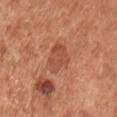No biopsy was performed on this lesion — it was imaged during a full skin examination and was not determined to be concerning. A region of skin cropped from a whole-body photographic capture, roughly 15 mm wide. Captured under white-light illumination. Measured at roughly 3.5 mm in maximum diameter. The total-body-photography lesion software estimated a mean CIELAB color near L≈51 a*≈27 b*≈34, roughly 8 lightness units darker than nearby skin, and a normalized lesion–skin contrast near 5.5. The software also gave radial color variation of about 1. On the chest. A male patient approximately 55 years of age.